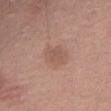location: the abdomen
patient: female, aged around 50
imaging modality: ~15 mm crop, total-body skin-cancer survey
illumination: white-light illumination
image-analysis metrics: an area of roughly 8 mm², an eccentricity of roughly 0.75, and a shape-asymmetry score of about 0.2 (0 = symmetric)
lesion size: ≈4.5 mm